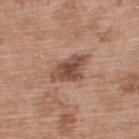Notes:
– workup: imaged on a skin check; not biopsied
– illumination: white-light illumination
– size: ≈5 mm
– image source: 15 mm crop, total-body photography
– location: the upper back
– patient: male, aged 48–52